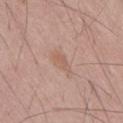Impression:
Captured during whole-body skin photography for melanoma surveillance; the lesion was not biopsied.
Acquisition and patient details:
The lesion's longest dimension is about 3 mm. The tile uses white-light illumination. Automated image analysis of the tile measured a border-irregularity rating of about 2.5/10, internal color variation of about 1.5 on a 0–10 scale, and peripheral color asymmetry of about 0.5. A lesion tile, about 15 mm wide, cut from a 3D total-body photograph. A male subject, aged 53 to 57.